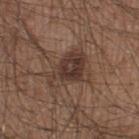Findings:
– notes · no biopsy performed (imaged during a skin exam)
– automated metrics · a lesion area of about 14 mm², a shape eccentricity near 0.75, and two-axis asymmetry of about 0.5; a nevus-likeness score of about 40/100 and a detector confidence of about 100 out of 100 that the crop contains a lesion
– lesion diameter · ~5 mm (longest diameter)
– subject · male, approximately 20 years of age
– imaging modality · ~15 mm crop, total-body skin-cancer survey
– lighting · white-light illumination
– site · the leg